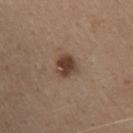{
  "patient": {
    "sex": "female",
    "age_approx": 35
  },
  "automated_metrics": {
    "area_mm2_approx": 5.5,
    "eccentricity": 0.5,
    "shape_asymmetry": 0.2,
    "cielab_L": 41,
    "cielab_a": 17,
    "cielab_b": 26,
    "vs_skin_contrast_norm": 10.0,
    "nevus_likeness_0_100": 90,
    "lesion_detection_confidence_0_100": 100
  },
  "site": "upper back",
  "image": {
    "source": "total-body photography crop",
    "field_of_view_mm": 15
  },
  "lighting": "white-light",
  "lesion_size": {
    "long_diameter_mm_approx": 3.0
  }
}